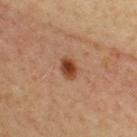The lesion was tiled from a total-body skin photograph and was not biopsied. A female patient, about 40 years old. The lesion is on the upper back. A roughly 15 mm field-of-view crop from a total-body skin photograph.